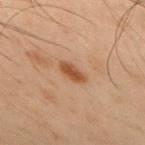Imaged during a routine full-body skin examination; the lesion was not biopsied and no histopathology is available.
The lesion is located on the upper back.
A male subject aged 48 to 52.
A lesion tile, about 15 mm wide, cut from a 3D total-body photograph.